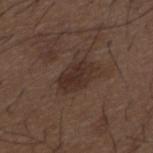follow-up=total-body-photography surveillance lesion; no biopsy
imaging modality=total-body-photography crop, ~15 mm field of view
lesion diameter=about 4 mm
anatomic site=the mid back
lighting=white-light
automated metrics=a border-irregularity index near 2/10, a within-lesion color-variation index near 2.5/10, and a peripheral color-asymmetry measure near 1; a nevus-likeness score of about 20/100
subject=male, aged approximately 50